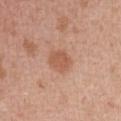Notes:
• follow-up — catalogued during a skin exam; not biopsied
• patient — female, in their 60s
• location — the front of the torso
• diameter — ≈3 mm
• tile lighting — white-light
• image source — total-body-photography crop, ~15 mm field of view
• automated lesion analysis — a footprint of about 7 mm²; a lesion color around L≈57 a*≈23 b*≈32 in CIELAB, roughly 8 lightness units darker than nearby skin, and a normalized border contrast of about 6.5; a border-irregularity index near 2/10 and a color-variation rating of about 1.5/10